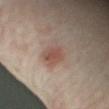<record>
  <biopsy_status>not biopsied; imaged during a skin examination</biopsy_status>
  <lesion_size>
    <long_diameter_mm_approx>3.0</long_diameter_mm_approx>
  </lesion_size>
  <site>right forearm</site>
  <patient>
    <sex>female</sex>
    <age_approx>35</age_approx>
  </patient>
  <lighting>cross-polarized</lighting>
  <image>
    <source>total-body photography crop</source>
    <field_of_view_mm>15</field_of_view_mm>
  </image>
</record>